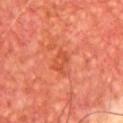Assessment: This lesion was catalogued during total-body skin photography and was not selected for biopsy. Context: The lesion is on the chest. Captured under cross-polarized illumination. Approximately 2.5 mm at its widest. A close-up tile cropped from a whole-body skin photograph, about 15 mm across. A male subject, approximately 65 years of age. The lesion-visualizer software estimated a mean CIELAB color near L≈48 a*≈34 b*≈37, about 7 CIELAB-L* units darker than the surrounding skin, and a lesion-to-skin contrast of about 5 (normalized; higher = more distinct). The analysis additionally found a nevus-likeness score of about 0/100 and a lesion-detection confidence of about 100/100.